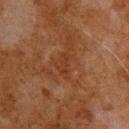| field | value |
|---|---|
| notes | catalogued during a skin exam; not biopsied |
| subject | male, approximately 80 years of age |
| imaging modality | total-body-photography crop, ~15 mm field of view |
| lighting | cross-polarized illumination |
| lesion diameter | ~2.5 mm (longest diameter) |
| anatomic site | the back |